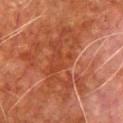Impression: The lesion was photographed on a routine skin check and not biopsied; there is no pathology result. Acquisition and patient details: Longest diameter approximately 9.5 mm. A lesion tile, about 15 mm wide, cut from a 3D total-body photograph. Captured under cross-polarized illumination. A male subject aged 78 to 82. The lesion is on the chest.